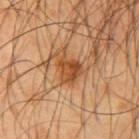follow-up: no biopsy performed (imaged during a skin exam) | tile lighting: cross-polarized illumination | TBP lesion metrics: an outline eccentricity of about 0.5 (0 = round, 1 = elongated) and a shape-asymmetry score of about 0.4 (0 = symmetric); an average lesion color of about L≈46 a*≈24 b*≈37 (CIELAB), roughly 10 lightness units darker than nearby skin, and a normalized lesion–skin contrast near 8; an automated nevus-likeness rating near 70 out of 100 and lesion-presence confidence of about 100/100 | body site: the arm | patient: male, roughly 45 years of age | acquisition: 15 mm crop, total-body photography.